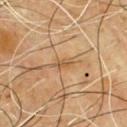biopsy status — total-body-photography surveillance lesion; no biopsy
subject — male, in their mid-60s
image source — total-body-photography crop, ~15 mm field of view
lighting — cross-polarized
location — the chest
size — about 3 mm